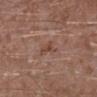This lesion was catalogued during total-body skin photography and was not selected for biopsy.
The patient is a male aged around 45.
The lesion is on the left lower leg.
A lesion tile, about 15 mm wide, cut from a 3D total-body photograph.